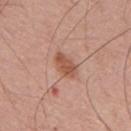Imaged during a routine full-body skin examination; the lesion was not biopsied and no histopathology is available.
The lesion is located on the mid back.
A roughly 15 mm field-of-view crop from a total-body skin photograph.
A male patient roughly 55 years of age.
Automated image analysis of the tile measured roughly 10 lightness units darker than nearby skin and a normalized lesion–skin contrast near 7.5.
The lesion's longest dimension is about 4 mm.